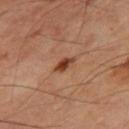Part of a total-body skin-imaging series; this lesion was reviewed on a skin check and was not flagged for biopsy. The lesion-visualizer software estimated an area of roughly 3.5 mm² and two-axis asymmetry of about 0.2. And it measured a border-irregularity rating of about 2/10 and a within-lesion color-variation index near 4.5/10. The analysis additionally found an automated nevus-likeness rating near 90 out of 100 and lesion-presence confidence of about 100/100. The tile uses cross-polarized illumination. On the right thigh. The lesion's longest dimension is about 3 mm. A male patient roughly 65 years of age. Cropped from a whole-body photographic skin survey; the tile spans about 15 mm.